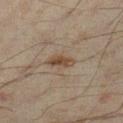Notes:
- notes: imaged on a skin check; not biopsied
- subject: male, approximately 45 years of age
- acquisition: 15 mm crop, total-body photography
- body site: the left lower leg